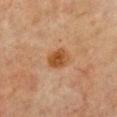Notes:
• subject — female, approximately 80 years of age
• location — the front of the torso
• image-analysis metrics — a footprint of about 5 mm², a shape eccentricity near 0.6, and a symmetry-axis asymmetry near 0.25; a border-irregularity index near 2/10, a color-variation rating of about 3/10, and peripheral color asymmetry of about 1
• lesion diameter — ~3 mm (longest diameter)
• image source — total-body-photography crop, ~15 mm field of view